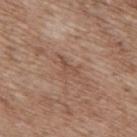The lesion was photographed on a routine skin check and not biopsied; there is no pathology result. A male subject aged approximately 70. The total-body-photography lesion software estimated an area of roughly 3 mm² and a symmetry-axis asymmetry near 0.6. The software also gave a border-irregularity index near 6/10 and peripheral color asymmetry of about 0. Approximately 3 mm at its widest. This image is a 15 mm lesion crop taken from a total-body photograph. From the upper back.